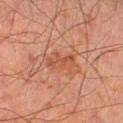Q: Was this lesion biopsied?
A: catalogued during a skin exam; not biopsied
Q: What is the imaging modality?
A: 15 mm crop, total-body photography
Q: Patient demographics?
A: male, in their mid-60s
Q: Automated lesion metrics?
A: an area of roughly 5 mm², an outline eccentricity of about 0.95 (0 = round, 1 = elongated), and two-axis asymmetry of about 0.35; a border-irregularity index near 5/10 and internal color variation of about 0.5 on a 0–10 scale; a nevus-likeness score of about 0/100
Q: Lesion size?
A: ~4 mm (longest diameter)
Q: Where on the body is the lesion?
A: the leg